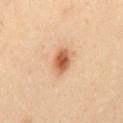Clinical summary:
The lesion's longest dimension is about 3 mm. Located on the back. Captured under cross-polarized illumination. A female patient, aged 28–32. A close-up tile cropped from a whole-body skin photograph, about 15 mm across. Automated image analysis of the tile measured an average lesion color of about L≈48 a*≈20 b*≈30 (CIELAB), about 12 CIELAB-L* units darker than the surrounding skin, and a normalized lesion–skin contrast near 9.